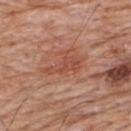<case>
  <patient>
    <sex>male</sex>
    <age_approx>80</age_approx>
  </patient>
  <image>
    <source>total-body photography crop</source>
    <field_of_view_mm>15</field_of_view_mm>
  </image>
  <site>upper back</site>
</case>